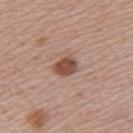workup — imaged on a skin check; not biopsied | patient — female, aged approximately 50 | acquisition — total-body-photography crop, ~15 mm field of view | site — the left upper arm.